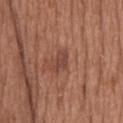No biopsy was performed on this lesion — it was imaged during a full skin examination and was not determined to be concerning. A close-up tile cropped from a whole-body skin photograph, about 15 mm across. The tile uses white-light illumination. The lesion is located on the head or neck. A male subject in their 40s. Approximately 3 mm at its widest.